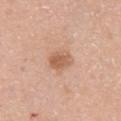Captured under white-light illumination. Automated image analysis of the tile measured a footprint of about 5.5 mm², an outline eccentricity of about 0.6 (0 = round, 1 = elongated), and a shape-asymmetry score of about 0.15 (0 = symmetric). The software also gave a nevus-likeness score of about 80/100 and lesion-presence confidence of about 100/100. A female subject approximately 40 years of age. On the right upper arm. A roughly 15 mm field-of-view crop from a total-body skin photograph. The lesion's longest dimension is about 3 mm.